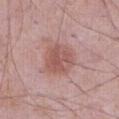Recorded during total-body skin imaging; not selected for excision or biopsy.
From the abdomen.
Measured at roughly 4 mm in maximum diameter.
Cropped from a whole-body photographic skin survey; the tile spans about 15 mm.
The subject is a male aged approximately 70.